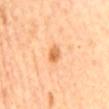notes=no biopsy performed (imaged during a skin exam); image=15 mm crop, total-body photography; anatomic site=the mid back; patient=female, aged 63–67.